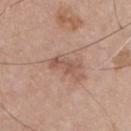workup: catalogued during a skin exam; not biopsied | patient: male, aged 73–77 | anatomic site: the chest | lighting: white-light illumination | lesion size: about 3.5 mm | imaging modality: total-body-photography crop, ~15 mm field of view.